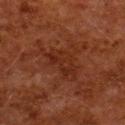On the upper back.
The patient is a female aged 48 to 52.
This is a cross-polarized tile.
Automated tile analysis of the lesion measured a lesion color around L≈22 a*≈22 b*≈26 in CIELAB, about 5 CIELAB-L* units darker than the surrounding skin, and a lesion-to-skin contrast of about 5.5 (normalized; higher = more distinct). It also reported a nevus-likeness score of about 0/100 and a lesion-detection confidence of about 100/100.
A roughly 15 mm field-of-view crop from a total-body skin photograph.